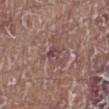Part of a total-body skin-imaging series; this lesion was reviewed on a skin check and was not flagged for biopsy.
Cropped from a whole-body photographic skin survey; the tile spans about 15 mm.
Imaged with white-light lighting.
The patient is a male aged 78–82.
On the left lower leg.
Measured at roughly 2.5 mm in maximum diameter.